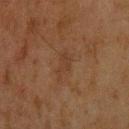biopsy status: catalogued during a skin exam; not biopsied | patient: male, aged around 45 | tile lighting: cross-polarized | acquisition: ~15 mm crop, total-body skin-cancer survey | location: the upper back | TBP lesion metrics: a shape-asymmetry score of about 0.35 (0 = symmetric).